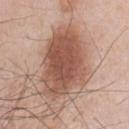Assessment:
No biopsy was performed on this lesion — it was imaged during a full skin examination and was not determined to be concerning.
Clinical summary:
Longest diameter approximately 9.5 mm. The patient is a male about 60 years old. A 15 mm close-up tile from a total-body photography series done for melanoma screening. The lesion is on the front of the torso.